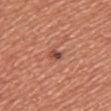Q: What did automated image analysis measure?
A: border irregularity of about 3 on a 0–10 scale, a within-lesion color-variation index near 4.5/10, and radial color variation of about 1.5; a nevus-likeness score of about 35/100 and a lesion-detection confidence of about 100/100
Q: Lesion size?
A: about 2 mm
Q: What is the anatomic site?
A: the chest
Q: What is the imaging modality?
A: ~15 mm crop, total-body skin-cancer survey
Q: What lighting was used for the tile?
A: white-light
Q: Patient demographics?
A: female, aged approximately 40